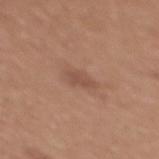Case summary:
- workup — catalogued during a skin exam; not biopsied
- imaging modality — 15 mm crop, total-body photography
- size — about 3 mm
- subject — female, in their mid- to late 30s
- automated lesion analysis — a footprint of about 4 mm², an outline eccentricity of about 0.85 (0 = round, 1 = elongated), and a shape-asymmetry score of about 0.35 (0 = symmetric); a lesion color around L≈50 a*≈20 b*≈28 in CIELAB; a nevus-likeness score of about 5/100 and a lesion-detection confidence of about 100/100
- site — the chest